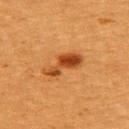- biopsy status: catalogued during a skin exam; not biopsied
- lesion diameter: ≈5 mm
- TBP lesion metrics: a mean CIELAB color near L≈40 a*≈27 b*≈40, a lesion–skin lightness drop of about 13, and a normalized border contrast of about 10
- patient: female, in their mid- to late 50s
- anatomic site: the upper back
- acquisition: total-body-photography crop, ~15 mm field of view
- tile lighting: cross-polarized illumination